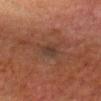Findings:
* workup — total-body-photography surveillance lesion; no biopsy
* illumination — cross-polarized
* imaging modality — 15 mm crop, total-body photography
* image-analysis metrics — a lesion area of about 4 mm², an eccentricity of roughly 0.65, and two-axis asymmetry of about 0.25; an average lesion color of about L≈28 a*≈14 b*≈20 (CIELAB), a lesion–skin lightness drop of about 5, and a normalized border contrast of about 6.5; a border-irregularity rating of about 2.5/10, internal color variation of about 3 on a 0–10 scale, and radial color variation of about 1
* lesion size — ≈2.5 mm
* subject — male, aged around 80
* body site — the head or neck